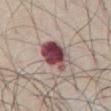Assessment:
No biopsy was performed on this lesion — it was imaged during a full skin examination and was not determined to be concerning.
Acquisition and patient details:
The subject is a male aged around 70. On the front of the torso. A close-up tile cropped from a whole-body skin photograph, about 15 mm across.